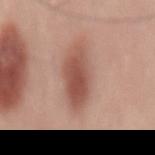Impression: The lesion was tiled from a total-body skin photograph and was not biopsied. Acquisition and patient details: The lesion is on the mid back. Cropped from a whole-body photographic skin survey; the tile spans about 15 mm. The lesion-visualizer software estimated a border-irregularity rating of about 3/10, a color-variation rating of about 4.5/10, and a peripheral color-asymmetry measure near 1.5. The analysis additionally found a nevus-likeness score of about 95/100 and a lesion-detection confidence of about 100/100. A female subject, aged 38–42. Imaged with white-light lighting. Longest diameter approximately 6.5 mm.